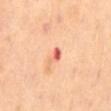Case summary:
- notes: imaged on a skin check; not biopsied
- anatomic site: the mid back
- subject: male, in their 70s
- size: ≈2.5 mm
- imaging modality: ~15 mm tile from a whole-body skin photo
- illumination: cross-polarized